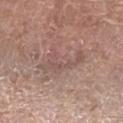The lesion was photographed on a routine skin check and not biopsied; there is no pathology result. Measured at roughly 5.5 mm in maximum diameter. Captured under white-light illumination. A 15 mm close-up tile from a total-body photography series done for melanoma screening. Automated tile analysis of the lesion measured a border-irregularity rating of about 8/10 and a peripheral color-asymmetry measure near 1. The analysis additionally found a nevus-likeness score of about 0/100 and a detector confidence of about 70 out of 100 that the crop contains a lesion. On the right upper arm. The subject is a male in their 60s.